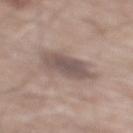| feature | finding |
|---|---|
| acquisition | ~15 mm tile from a whole-body skin photo |
| anatomic site | the mid back |
| diameter | ≈5 mm |
| subject | male, approximately 60 years of age |
| automated lesion analysis | a lesion area of about 13 mm², a shape eccentricity near 0.75, and two-axis asymmetry of about 0.2; an average lesion color of about L≈52 a*≈14 b*≈19 (CIELAB), about 10 CIELAB-L* units darker than the surrounding skin, and a lesion-to-skin contrast of about 8 (normalized; higher = more distinct); a border-irregularity index near 2.5/10, internal color variation of about 3 on a 0–10 scale, and radial color variation of about 1; a classifier nevus-likeness of about 15/100 and a detector confidence of about 100 out of 100 that the crop contains a lesion |
| lighting | white-light illumination |